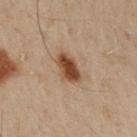follow-up: catalogued during a skin exam; not biopsied
site: the left arm
patient: male, in their 50s
image: ~15 mm tile from a whole-body skin photo